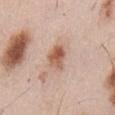The lesion was photographed on a routine skin check and not biopsied; there is no pathology result.
From the abdomen.
This image is a 15 mm lesion crop taken from a total-body photograph.
A male patient aged 53–57.